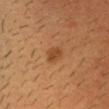notes = catalogued during a skin exam; not biopsied | patient = female, in their 50s | image source = ~15 mm crop, total-body skin-cancer survey | tile lighting = cross-polarized | lesion size = ~2.5 mm (longest diameter).